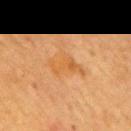Findings:
– automated metrics — an average lesion color of about L≈49 a*≈20 b*≈39 (CIELAB), roughly 5 lightness units darker than nearby skin, and a normalized lesion–skin contrast near 5.5
– lesion diameter — ~4.5 mm (longest diameter)
– tile lighting — cross-polarized
– location — the back
– imaging modality — total-body-photography crop, ~15 mm field of view
– subject — female, in their mid- to late 50s